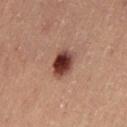biopsy status = imaged on a skin check; not biopsied
illumination = cross-polarized
subject = female, roughly 45 years of age
body site = the left thigh
image = ~15 mm tile from a whole-body skin photo